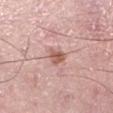This lesion was catalogued during total-body skin photography and was not selected for biopsy. The lesion is located on the left lower leg. The patient is a male approximately 50 years of age. This is a white-light tile. Longest diameter approximately 2.5 mm. A 15 mm crop from a total-body photograph taken for skin-cancer surveillance.